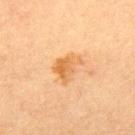Assessment: The lesion was photographed on a routine skin check and not biopsied; there is no pathology result. Background: Located on the upper back. This image is a 15 mm lesion crop taken from a total-body photograph. The recorded lesion diameter is about 3.5 mm. The tile uses cross-polarized illumination. The patient is a male aged approximately 60.